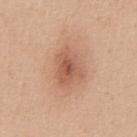Assessment:
Captured during whole-body skin photography for melanoma surveillance; the lesion was not biopsied.
Background:
Cropped from a total-body skin-imaging series; the visible field is about 15 mm. The lesion is on the abdomen. About 3.5 mm across. Imaged with white-light lighting. Automated tile analysis of the lesion measured an area of roughly 8.5 mm² and an eccentricity of roughly 0.7. And it measured a mean CIELAB color near L≈57 a*≈24 b*≈32 and about 10 CIELAB-L* units darker than the surrounding skin. The analysis additionally found a classifier nevus-likeness of about 45/100 and lesion-presence confidence of about 100/100. A male patient roughly 50 years of age.